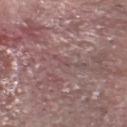workup = no biopsy performed (imaged during a skin exam)
lighting = white-light illumination
patient = male, aged approximately 85 or older
image = total-body-photography crop, ~15 mm field of view
body site = the head or neck
automated metrics = a lesion area of about 1 mm², a shape eccentricity near 0.9, and two-axis asymmetry of about 0.4; a border-irregularity rating of about 3.5/10 and internal color variation of about 0 on a 0–10 scale